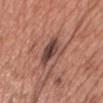Clinical impression:
No biopsy was performed on this lesion — it was imaged during a full skin examination and was not determined to be concerning.
Background:
About 5.5 mm across. Imaged with white-light lighting. A 15 mm crop from a total-body photograph taken for skin-cancer surveillance. The subject is a male about 40 years old. The lesion is located on the head or neck.